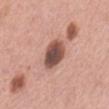Impression:
Part of a total-body skin-imaging series; this lesion was reviewed on a skin check and was not flagged for biopsy.
Image and clinical context:
A female patient, in their mid- to late 60s. A close-up tile cropped from a whole-body skin photograph, about 15 mm across. Imaged with white-light lighting. Longest diameter approximately 4 mm. Located on the abdomen.